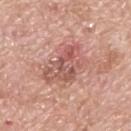Part of a total-body skin-imaging series; this lesion was reviewed on a skin check and was not flagged for biopsy.
Measured at roughly 6 mm in maximum diameter.
Captured under white-light illumination.
The subject is a male roughly 70 years of age.
Located on the upper back.
A close-up tile cropped from a whole-body skin photograph, about 15 mm across.